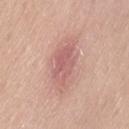notes: imaged on a skin check; not biopsied
automated metrics: a lesion area of about 16 mm² and a shape eccentricity near 0.85; a lesion color around L≈61 a*≈24 b*≈24 in CIELAB, roughly 9 lightness units darker than nearby skin, and a normalized border contrast of about 6; a border-irregularity rating of about 3/10 and a within-lesion color-variation index near 3.5/10
patient: female, in their mid-60s
lighting: white-light
image: 15 mm crop, total-body photography
body site: the lower back
lesion diameter: ≈6 mm